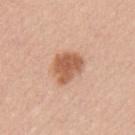Recorded during total-body skin imaging; not selected for excision or biopsy.
The subject is a female approximately 40 years of age.
The lesion is on the left upper arm.
The lesion-visualizer software estimated an area of roughly 10 mm², an eccentricity of roughly 0.65, and two-axis asymmetry of about 0.25. The analysis additionally found a lesion color around L≈59 a*≈23 b*≈34 in CIELAB, roughly 13 lightness units darker than nearby skin, and a lesion-to-skin contrast of about 9 (normalized; higher = more distinct). It also reported border irregularity of about 2.5 on a 0–10 scale, a color-variation rating of about 3/10, and a peripheral color-asymmetry measure near 1. And it measured a nevus-likeness score of about 90/100 and a detector confidence of about 100 out of 100 that the crop contains a lesion.
The lesion's longest dimension is about 4 mm.
This is a white-light tile.
Cropped from a whole-body photographic skin survey; the tile spans about 15 mm.